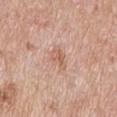Findings:
– biopsy status: catalogued during a skin exam; not biopsied
– subject: male, about 60 years old
– size: ≈3 mm
– acquisition: ~15 mm crop, total-body skin-cancer survey
– image-analysis metrics: a footprint of about 4.5 mm², a shape eccentricity near 0.8, and a shape-asymmetry score of about 0.25 (0 = symmetric); a lesion–skin lightness drop of about 8 and a lesion-to-skin contrast of about 5.5 (normalized; higher = more distinct); a within-lesion color-variation index near 3/10; a detector confidence of about 100 out of 100 that the crop contains a lesion
– anatomic site: the mid back